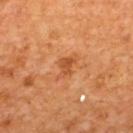Imaged during a routine full-body skin examination; the lesion was not biopsied and no histopathology is available. The patient is a male approximately 65 years of age. Approximately 3 mm at its widest. The total-body-photography lesion software estimated a mean CIELAB color near L≈50 a*≈28 b*≈41, roughly 9 lightness units darker than nearby skin, and a normalized lesion–skin contrast near 6.5. It also reported a border-irregularity index near 3.5/10 and a within-lesion color-variation index near 2/10. The lesion is on the upper back. A 15 mm close-up tile from a total-body photography series done for melanoma screening.